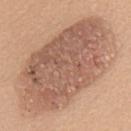The lesion was tiled from a total-body skin photograph and was not biopsied. A close-up tile cropped from a whole-body skin photograph, about 15 mm across. The lesion is located on the back. Longest diameter approximately 14 mm. This is a white-light tile. A male patient in their 60s.